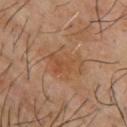Captured during whole-body skin photography for melanoma surveillance; the lesion was not biopsied.
The lesion is located on the upper back.
Approximately 6 mm at its widest.
Imaged with cross-polarized lighting.
The subject is a male aged 63–67.
Cropped from a whole-body photographic skin survey; the tile spans about 15 mm.